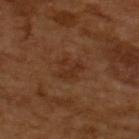workup: imaged on a skin check; not biopsied
acquisition: total-body-photography crop, ~15 mm field of view
lighting: cross-polarized illumination
TBP lesion metrics: a lesion area of about 7 mm² and an outline eccentricity of about 0.4 (0 = round, 1 = elongated); a nevus-likeness score of about 0/100 and a lesion-detection confidence of about 100/100
patient: male, approximately 65 years of age